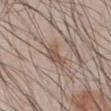follow-up: catalogued during a skin exam; not biopsied | body site: the abdomen | lesion diameter: about 3 mm | patient: male, about 55 years old | image: total-body-photography crop, ~15 mm field of view | automated lesion analysis: an area of roughly 5 mm², an outline eccentricity of about 0.75 (0 = round, 1 = elongated), and a symmetry-axis asymmetry near 0.4.